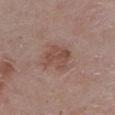Case summary:
• biopsy status: catalogued during a skin exam; not biopsied
• body site: the chest
• patient: female, roughly 50 years of age
• acquisition: ~15 mm tile from a whole-body skin photo
• tile lighting: white-light illumination
• automated metrics: a lesion area of about 9.5 mm², an outline eccentricity of about 0.35 (0 = round, 1 = elongated), and a shape-asymmetry score of about 0.2 (0 = symmetric); a classifier nevus-likeness of about 15/100 and lesion-presence confidence of about 100/100
• size: about 3.5 mm